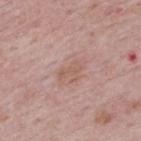Assessment: Imaged during a routine full-body skin examination; the lesion was not biopsied and no histopathology is available. Acquisition and patient details: The total-body-photography lesion software estimated a border-irregularity rating of about 5.5/10, a color-variation rating of about 0/10, and peripheral color asymmetry of about 0. The software also gave a nevus-likeness score of about 0/100 and lesion-presence confidence of about 100/100. A 15 mm crop from a total-body photograph taken for skin-cancer surveillance. Located on the upper back. Imaged with white-light lighting. The recorded lesion diameter is about 3 mm. The patient is a female aged approximately 50.